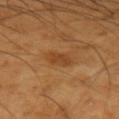The lesion was photographed on a routine skin check and not biopsied; there is no pathology result. A close-up tile cropped from a whole-body skin photograph, about 15 mm across. A male subject roughly 60 years of age. The lesion's longest dimension is about 3.5 mm. The tile uses cross-polarized illumination. On the right upper arm.